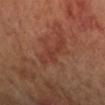Captured during whole-body skin photography for melanoma surveillance; the lesion was not biopsied. Cropped from a whole-body photographic skin survey; the tile spans about 15 mm. This is a cross-polarized tile. Longest diameter approximately 5.5 mm. A female patient roughly 65 years of age. Located on the left arm.